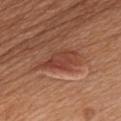| key | value |
|---|---|
| follow-up | imaged on a skin check; not biopsied |
| subject | male, aged 58 to 62 |
| image source | ~15 mm tile from a whole-body skin photo |
| anatomic site | the chest |
| lesion diameter | ~5.5 mm (longest diameter) |
| lighting | white-light |
| automated metrics | border irregularity of about 4.5 on a 0–10 scale and internal color variation of about 3.5 on a 0–10 scale |